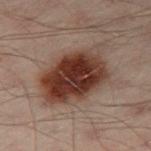Part of a total-body skin-imaging series; this lesion was reviewed on a skin check and was not flagged for biopsy. The subject is a male aged around 50. A region of skin cropped from a whole-body photographic capture, roughly 15 mm wide. Located on the leg.